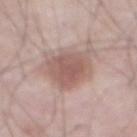* image — total-body-photography crop, ~15 mm field of view
* illumination — white-light
* TBP lesion metrics — an area of roughly 16 mm² and a symmetry-axis asymmetry near 0.2; a border-irregularity index near 2.5/10, a within-lesion color-variation index near 3.5/10, and a peripheral color-asymmetry measure near 1
* subject — male, in their mid- to late 70s
* site — the abdomen